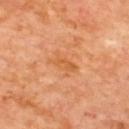Assessment: Part of a total-body skin-imaging series; this lesion was reviewed on a skin check and was not flagged for biopsy. Acquisition and patient details: A male patient, in their 60s. A 15 mm close-up tile from a total-body photography series done for melanoma screening. On the back.